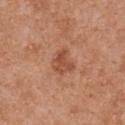Context: A 15 mm close-up extracted from a 3D total-body photography capture. Captured under white-light illumination. Located on the upper back. The recorded lesion diameter is about 3 mm. A female subject, aged 38 to 42.